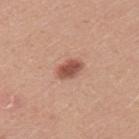{
  "lighting": "white-light",
  "site": "left upper arm",
  "image": {
    "source": "total-body photography crop",
    "field_of_view_mm": 15
  },
  "automated_metrics": {
    "area_mm2_approx": 5.0,
    "eccentricity": 0.8,
    "shape_asymmetry": 0.2,
    "nevus_likeness_0_100": 95,
    "lesion_detection_confidence_0_100": 100
  },
  "patient": {
    "sex": "male",
    "age_approx": 25
  },
  "diagnosis": {
    "histopathology": "dysplastic (Clark) nevus",
    "malignancy": "benign",
    "taxonomic_path": [
      "Benign",
      "Benign melanocytic proliferations",
      "Nevus",
      "Nevus, Atypical, Dysplastic, or Clark"
    ]
  }
}